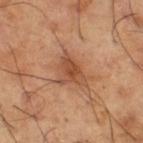Assessment: The lesion was photographed on a routine skin check and not biopsied; there is no pathology result. Background: The subject is a male aged 63 to 67. A roughly 15 mm field-of-view crop from a total-body skin photograph. Imaged with cross-polarized lighting. About 4.5 mm across. On the leg.